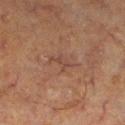Assessment: Imaged during a routine full-body skin examination; the lesion was not biopsied and no histopathology is available. Background: A male patient, aged 63 to 67. The tile uses cross-polarized illumination. On the right lower leg. Longest diameter approximately 2.5 mm. This image is a 15 mm lesion crop taken from a total-body photograph.